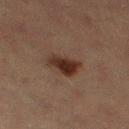This lesion was catalogued during total-body skin photography and was not selected for biopsy. Cropped from a total-body skin-imaging series; the visible field is about 15 mm. The lesion is on the right thigh. A female subject aged 53–57. Captured under cross-polarized illumination. The recorded lesion diameter is about 4 mm.